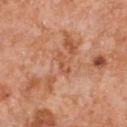Recorded during total-body skin imaging; not selected for excision or biopsy.
Measured at roughly 3 mm in maximum diameter.
The patient is a male about 55 years old.
The lesion is located on the chest.
A 15 mm close-up tile from a total-body photography series done for melanoma screening.
Automated tile analysis of the lesion measured a border-irregularity rating of about 4/10 and radial color variation of about 0.5.